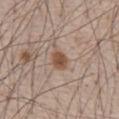workup: imaged on a skin check; not biopsied
image: 15 mm crop, total-body photography
body site: the front of the torso
illumination: white-light illumination
subject: male, aged 63 to 67
size: about 3 mm
automated lesion analysis: a footprint of about 5 mm², an outline eccentricity of about 0.7 (0 = round, 1 = elongated), and a shape-asymmetry score of about 0.25 (0 = symmetric); a border-irregularity rating of about 2/10, a color-variation rating of about 2.5/10, and radial color variation of about 1; an automated nevus-likeness rating near 95 out of 100 and lesion-presence confidence of about 100/100